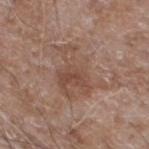Case summary:
* follow-up · no biopsy performed (imaged during a skin exam)
* image source · ~15 mm tile from a whole-body skin photo
* subject · male, roughly 60 years of age
* lighting · white-light
* body site · the left lower leg
* lesion size · ~6.5 mm (longest diameter)